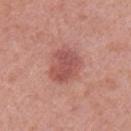Case summary:
- biopsy status: catalogued during a skin exam; not biopsied
- image source: 15 mm crop, total-body photography
- subject: female, approximately 40 years of age
- illumination: white-light
- anatomic site: the left upper arm
- lesion diameter: ≈4.5 mm